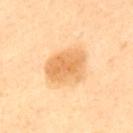Assessment: Recorded during total-body skin imaging; not selected for excision or biopsy. Acquisition and patient details: A 15 mm close-up tile from a total-body photography series done for melanoma screening. Located on the mid back. The recorded lesion diameter is about 5 mm. Imaged with cross-polarized lighting. The lesion-visualizer software estimated a lesion area of about 15 mm² and a shape-asymmetry score of about 0.15 (0 = symmetric). And it measured an average lesion color of about L≈70 a*≈22 b*≈44 (CIELAB) and a normalized lesion–skin contrast near 7.5. The analysis additionally found a border-irregularity rating of about 1.5/10, a color-variation rating of about 4.5/10, and radial color variation of about 1.5. It also reported an automated nevus-likeness rating near 50 out of 100 and a detector confidence of about 100 out of 100 that the crop contains a lesion. The patient is a male aged approximately 60.